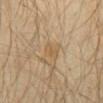Case summary:
* notes: no biopsy performed (imaged during a skin exam)
* site: the abdomen
* patient: male, aged 28 to 32
* lesion diameter: ≈2.5 mm
* tile lighting: cross-polarized
* acquisition: ~15 mm crop, total-body skin-cancer survey